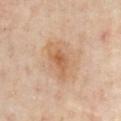Imaged during a routine full-body skin examination; the lesion was not biopsied and no histopathology is available. A close-up tile cropped from a whole-body skin photograph, about 15 mm across. A male subject, in their mid- to late 60s. On the chest. Imaged with cross-polarized lighting. About 5.5 mm across.